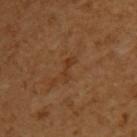Assessment: Recorded during total-body skin imaging; not selected for excision or biopsy. Acquisition and patient details: A region of skin cropped from a whole-body photographic capture, roughly 15 mm wide. The tile uses cross-polarized illumination. Measured at roughly 3 mm in maximum diameter. On the left upper arm. The subject is a female aged approximately 55. The lesion-visualizer software estimated an area of roughly 2.5 mm² and a symmetry-axis asymmetry near 0.45. And it measured an average lesion color of about L≈37 a*≈22 b*≈34 (CIELAB) and a lesion–skin lightness drop of about 6.